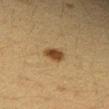No biopsy was performed on this lesion — it was imaged during a full skin examination and was not determined to be concerning.
A close-up tile cropped from a whole-body skin photograph, about 15 mm across.
Approximately 2.5 mm at its widest.
A female patient aged around 30.
From the right forearm.
An algorithmic analysis of the crop reported border irregularity of about 1.5 on a 0–10 scale, internal color variation of about 2 on a 0–10 scale, and radial color variation of about 0.5.